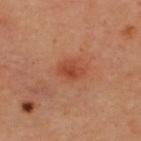<record>
<biopsy_status>not biopsied; imaged during a skin examination</biopsy_status>
<lesion_size>
  <long_diameter_mm_approx>3.0</long_diameter_mm_approx>
</lesion_size>
<site>upper back</site>
<automated_metrics>
  <cielab_L>46</cielab_L>
  <cielab_a>30</cielab_a>
  <cielab_b>35</cielab_b>
  <vs_skin_darker_L>9.0</vs_skin_darker_L>
</automated_metrics>
<patient>
  <sex>female</sex>
  <age_approx>45</age_approx>
</patient>
<image>
  <source>total-body photography crop</source>
  <field_of_view_mm>15</field_of_view_mm>
</image>
</record>